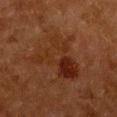<case>
<biopsy_status>not biopsied; imaged during a skin examination</biopsy_status>
<lesion_size>
  <long_diameter_mm_approx>7.0</long_diameter_mm_approx>
</lesion_size>
<automated_metrics>
  <area_mm2_approx>21.0</area_mm2_approx>
  <eccentricity>0.6</eccentricity>
  <shape_asymmetry>0.5</shape_asymmetry>
  <cielab_L>23</cielab_L>
  <cielab_a>19</cielab_a>
  <cielab_b>26</cielab_b>
  <vs_skin_darker_L>6.0</vs_skin_darker_L>
  <vs_skin_contrast_norm>6.5</vs_skin_contrast_norm>
  <color_variation_0_10>8.5</color_variation_0_10>
  <peripheral_color_asymmetry>3.0</peripheral_color_asymmetry>
  <nevus_likeness_0_100>0</nevus_likeness_0_100>
</automated_metrics>
<image>
  <source>total-body photography crop</source>
  <field_of_view_mm>15</field_of_view_mm>
</image>
<patient>
  <sex>female</sex>
  <age_approx>50</age_approx>
</patient>
<site>chest</site>
</case>